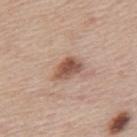* biopsy status — imaged on a skin check; not biopsied
* automated metrics — border irregularity of about 2.5 on a 0–10 scale and radial color variation of about 1.5; an automated nevus-likeness rating near 90 out of 100
* body site — the mid back
* image source — 15 mm crop, total-body photography
* tile lighting — white-light illumination
* subject — male, approximately 45 years of age
* lesion diameter — about 3.5 mm